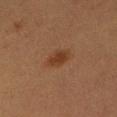| key | value |
|---|---|
| workup | catalogued during a skin exam; not biopsied |
| subject | female, in their 40s |
| body site | the left lower leg |
| image | ~15 mm crop, total-body skin-cancer survey |